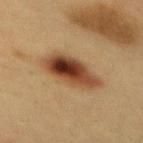Q: Was this lesion biopsied?
A: total-body-photography surveillance lesion; no biopsy
Q: How was this image acquired?
A: total-body-photography crop, ~15 mm field of view
Q: What is the lesion's diameter?
A: ~6.5 mm (longest diameter)
Q: Where on the body is the lesion?
A: the mid back
Q: Illumination type?
A: cross-polarized
Q: What did automated image analysis measure?
A: a lesion area of about 16 mm², a shape eccentricity near 0.9, and a symmetry-axis asymmetry near 0.2; a mean CIELAB color near L≈37 a*≈19 b*≈29, roughly 15 lightness units darker than nearby skin, and a normalized lesion–skin contrast near 12.5; a border-irregularity rating of about 2.5/10 and internal color variation of about 10 on a 0–10 scale; a classifier nevus-likeness of about 95/100 and a detector confidence of about 100 out of 100 that the crop contains a lesion
Q: Who is the patient?
A: female, aged 38 to 42